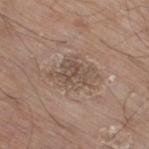No biopsy was performed on this lesion — it was imaged during a full skin examination and was not determined to be concerning.
Located on the right thigh.
A male subject aged 78 to 82.
A region of skin cropped from a whole-body photographic capture, roughly 15 mm wide.